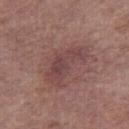Findings:
– imaging modality · 15 mm crop, total-body photography
– TBP lesion metrics · a lesion color around L≈44 a*≈21 b*≈19 in CIELAB and a normalized border contrast of about 6; a lesion-detection confidence of about 100/100
– subject · female, aged around 65
– location · the leg
– lesion size · ≈6 mm